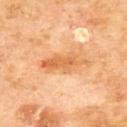notes: catalogued during a skin exam; not biopsied | illumination: cross-polarized | automated lesion analysis: a lesion area of about 10 mm², an eccentricity of roughly 0.9, and a shape-asymmetry score of about 0.25 (0 = symmetric); a nevus-likeness score of about 0/100 and a detector confidence of about 100 out of 100 that the crop contains a lesion | lesion size: ≈6 mm | site: the upper back | patient: male, roughly 70 years of age | image source: ~15 mm tile from a whole-body skin photo.